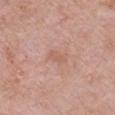This lesion was catalogued during total-body skin photography and was not selected for biopsy.
Located on the chest.
Captured under white-light illumination.
About 2.5 mm across.
The patient is a female aged around 70.
This image is a 15 mm lesion crop taken from a total-body photograph.